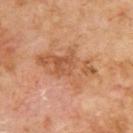Q: How large is the lesion?
A: about 7 mm
Q: How was this image acquired?
A: ~15 mm tile from a whole-body skin photo
Q: Where on the body is the lesion?
A: the upper back
Q: What lighting was used for the tile?
A: cross-polarized illumination
Q: Patient demographics?
A: male, about 70 years old